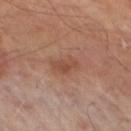TBP lesion metrics = an eccentricity of roughly 0.75; roughly 7 lightness units darker than nearby skin and a normalized lesion–skin contrast near 6; a color-variation rating of about 2/10 and radial color variation of about 0.5; a classifier nevus-likeness of about 0/100 and a detector confidence of about 100 out of 100 that the crop contains a lesion
subject = male, in their 70s
body site = the right thigh
imaging modality = ~15 mm crop, total-body skin-cancer survey
size = ~3.5 mm (longest diameter)
tile lighting = cross-polarized